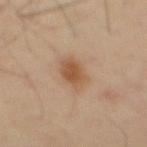This lesion was catalogued during total-body skin photography and was not selected for biopsy. A 15 mm close-up extracted from a 3D total-body photography capture. A male subject, aged around 70. Located on the abdomen. The tile uses cross-polarized illumination. The lesion's longest dimension is about 4 mm.